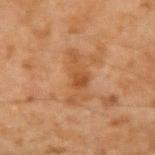lighting: cross-polarized illumination; patient: male, in their mid-40s; image: total-body-photography crop, ~15 mm field of view; location: the arm; lesion size: about 5.5 mm.